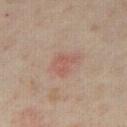Imaged during a routine full-body skin examination; the lesion was not biopsied and no histopathology is available.
A female subject, approximately 35 years of age.
A roughly 15 mm field-of-view crop from a total-body skin photograph.
From the abdomen.